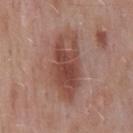<tbp_lesion>
<biopsy_status>not biopsied; imaged during a skin examination</biopsy_status>
<patient>
  <sex>male</sex>
  <age_approx>55</age_approx>
</patient>
<site>mid back</site>
<lighting>white-light</lighting>
<image>
  <source>total-body photography crop</source>
  <field_of_view_mm>15</field_of_view_mm>
</image>
</tbp_lesion>